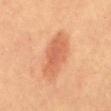<case>
<site>mid back</site>
<image>
  <source>total-body photography crop</source>
  <field_of_view_mm>15</field_of_view_mm>
</image>
<lesion_size>
  <long_diameter_mm_approx>6.5</long_diameter_mm_approx>
</lesion_size>
<patient>
  <sex>female</sex>
  <age_approx>60</age_approx>
</patient>
<lighting>cross-polarized</lighting>
</case>